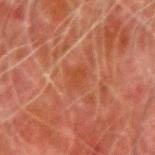Findings:
– subject — male, approximately 80 years of age
– tile lighting — cross-polarized illumination
– image-analysis metrics — lesion-presence confidence of about 95/100
– image — 15 mm crop, total-body photography
– lesion size — ~3 mm (longest diameter)
– anatomic site — the left forearm